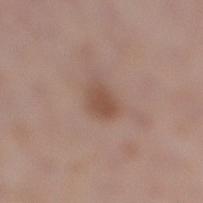Findings:
• workup — imaged on a skin check; not biopsied
• acquisition — total-body-photography crop, ~15 mm field of view
• lesion size — ≈2.5 mm
• subject — male, about 70 years old
• illumination — white-light illumination
• body site — the right lower leg